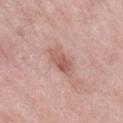Recorded during total-body skin imaging; not selected for excision or biopsy.
A female subject, aged approximately 70.
A region of skin cropped from a whole-body photographic capture, roughly 15 mm wide.
Longest diameter approximately 4.5 mm.
On the right lower leg.
Imaged with white-light lighting.